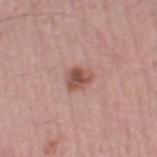The lesion was tiled from a total-body skin photograph and was not biopsied.
A male subject roughly 50 years of age.
Cropped from a whole-body photographic skin survey; the tile spans about 15 mm.
Captured under white-light illumination.
Located on the right thigh.
Automated image analysis of the tile measured border irregularity of about 2.5 on a 0–10 scale, a within-lesion color-variation index near 3.5/10, and peripheral color asymmetry of about 1.
Measured at roughly 2.5 mm in maximum diameter.